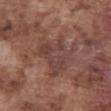Clinical summary:
A male patient approximately 75 years of age. On the abdomen. Automated image analysis of the tile measured an area of roughly 17 mm², an outline eccentricity of about 0.6 (0 = round, 1 = elongated), and a shape-asymmetry score of about 0.45 (0 = symmetric). And it measured border irregularity of about 6 on a 0–10 scale and a peripheral color-asymmetry measure near 1. Imaged with white-light lighting. A 15 mm crop from a total-body photograph taken for skin-cancer surveillance. Longest diameter approximately 6 mm.